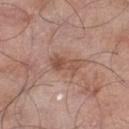{
  "biopsy_status": "not biopsied; imaged during a skin examination"
}